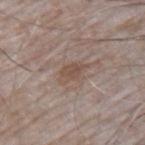Notes:
– follow-up · no biopsy performed (imaged during a skin exam)
– image · ~15 mm crop, total-body skin-cancer survey
– illumination · white-light illumination
– diameter · about 3.5 mm
– body site · the left upper arm
– patient · male, roughly 65 years of age